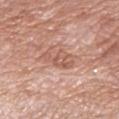Clinical impression: Recorded during total-body skin imaging; not selected for excision or biopsy. Background: Located on the right forearm. The subject is a female roughly 70 years of age. Cropped from a total-body skin-imaging series; the visible field is about 15 mm. Longest diameter approximately 4 mm.